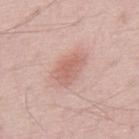A roughly 15 mm field-of-view crop from a total-body skin photograph.
The lesion is located on the mid back.
An algorithmic analysis of the crop reported a footprint of about 9 mm², an eccentricity of roughly 0.8, and two-axis asymmetry of about 0.25. It also reported a mean CIELAB color near L≈63 a*≈23 b*≈26 and a normalized border contrast of about 6.
The patient is a male in their 70s.